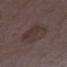Impression:
The lesion was photographed on a routine skin check and not biopsied; there is no pathology result.
Image and clinical context:
The lesion's longest dimension is about 5 mm. A female patient, aged 33–37. From the right lower leg. Imaged with white-light lighting. A roughly 15 mm field-of-view crop from a total-body skin photograph. Automated tile analysis of the lesion measured an automated nevus-likeness rating near 0 out of 100.